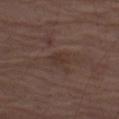workup = no biopsy performed (imaged during a skin exam)
tile lighting = white-light illumination
lesion diameter = ~3 mm (longest diameter)
body site = the left thigh
TBP lesion metrics = roughly 5 lightness units darker than nearby skin; a border-irregularity index near 2/10, a color-variation rating of about 2/10, and a peripheral color-asymmetry measure near 0.5; an automated nevus-likeness rating near 0 out of 100 and a detector confidence of about 100 out of 100 that the crop contains a lesion
acquisition = total-body-photography crop, ~15 mm field of view
subject = male, in their mid- to late 70s